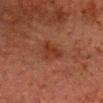Q: Was a biopsy performed?
A: no biopsy performed (imaged during a skin exam)
Q: Who is the patient?
A: male, aged around 80
Q: What is the lesion's diameter?
A: ≈3.5 mm
Q: Where on the body is the lesion?
A: the head or neck
Q: What is the imaging modality?
A: total-body-photography crop, ~15 mm field of view